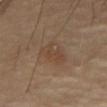notes = catalogued during a skin exam; not biopsied
subject = female, roughly 70 years of age
lesion size = ≈4.5 mm
site = the abdomen
illumination = cross-polarized
image = ~15 mm tile from a whole-body skin photo